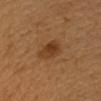Case summary:
- biopsy status · no biopsy performed (imaged during a skin exam)
- imaging modality · ~15 mm crop, total-body skin-cancer survey
- TBP lesion metrics · a lesion–skin lightness drop of about 9 and a normalized lesion–skin contrast near 8
- subject · female, about 45 years old
- illumination · cross-polarized illumination
- location · the arm